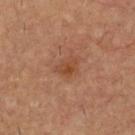Findings:
- workup · catalogued during a skin exam; not biopsied
- size · about 2.5 mm
- lighting · cross-polarized
- site · the upper back
- image · ~15 mm tile from a whole-body skin photo
- patient · male, in their mid- to late 60s